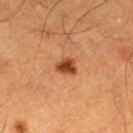No biopsy was performed on this lesion — it was imaged during a full skin examination and was not determined to be concerning.
The subject is a male roughly 60 years of age.
A 15 mm close-up extracted from a 3D total-body photography capture.
The lesion is located on the left thigh.
Longest diameter approximately 2.5 mm.
Captured under cross-polarized illumination.